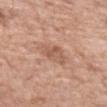Q: Was a biopsy performed?
A: total-body-photography surveillance lesion; no biopsy
Q: Automated lesion metrics?
A: border irregularity of about 3 on a 0–10 scale, a within-lesion color-variation index near 3.5/10, and peripheral color asymmetry of about 1; a classifier nevus-likeness of about 0/100 and lesion-presence confidence of about 100/100
Q: Lesion size?
A: about 4 mm
Q: Patient demographics?
A: female, roughly 70 years of age
Q: What lighting was used for the tile?
A: white-light illumination
Q: What kind of image is this?
A: total-body-photography crop, ~15 mm field of view
Q: What is the anatomic site?
A: the left forearm